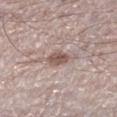Part of a total-body skin-imaging series; this lesion was reviewed on a skin check and was not flagged for biopsy.
This is a white-light tile.
The lesion's longest dimension is about 3 mm.
A 15 mm crop from a total-body photograph taken for skin-cancer surveillance.
A male patient aged around 50.
From the leg.
The total-body-photography lesion software estimated a border-irregularity index near 2/10, a color-variation rating of about 3.5/10, and a peripheral color-asymmetry measure near 1.5. And it measured a detector confidence of about 95 out of 100 that the crop contains a lesion.